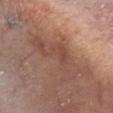<lesion>
  <automated_metrics>
    <area_mm2_approx>16.0</area_mm2_approx>
    <eccentricity>0.85</eccentricity>
    <shape_asymmetry>0.6</shape_asymmetry>
    <cielab_L>48</cielab_L>
    <cielab_a>21</cielab_a>
    <cielab_b>26</cielab_b>
    <vs_skin_darker_L>7.0</vs_skin_darker_L>
    <vs_skin_contrast_norm>5.5</vs_skin_contrast_norm>
  </automated_metrics>
  <image>
    <source>total-body photography crop</source>
    <field_of_view_mm>15</field_of_view_mm>
  </image>
  <lesion_size>
    <long_diameter_mm_approx>7.0</long_diameter_mm_approx>
  </lesion_size>
  <site>chest</site>
  <patient>
    <sex>male</sex>
    <age_approx>65</age_approx>
  </patient>
</lesion>